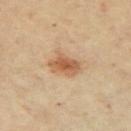<case>
<biopsy_status>not biopsied; imaged during a skin examination</biopsy_status>
<image>
  <source>total-body photography crop</source>
  <field_of_view_mm>15</field_of_view_mm>
</image>
<lighting>cross-polarized</lighting>
<patient>
  <sex>male</sex>
  <age_approx>65</age_approx>
</patient>
<site>right upper arm</site>
<automated_metrics>
  <border_irregularity_0_10>3.0</border_irregularity_0_10>
  <color_variation_0_10>4.0</color_variation_0_10>
  <peripheral_color_asymmetry>1.0</peripheral_color_asymmetry>
</automated_metrics>
<lesion_size>
  <long_diameter_mm_approx>3.5</long_diameter_mm_approx>
</lesion_size>
</case>